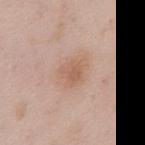Recorded during total-body skin imaging; not selected for excision or biopsy. A male subject aged around 55. A close-up tile cropped from a whole-body skin photograph, about 15 mm across. The lesion is on the chest.